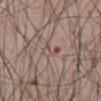Imaged during a routine full-body skin examination; the lesion was not biopsied and no histopathology is available.
This image is a 15 mm lesion crop taken from a total-body photograph.
The tile uses white-light illumination.
The recorded lesion diameter is about 3 mm.
A male patient in their 70s.
Located on the front of the torso.